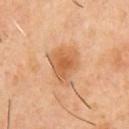- acquisition: ~15 mm tile from a whole-body skin photo
- patient: male, aged around 55
- illumination: cross-polarized
- site: the chest